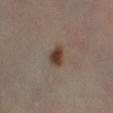The total-body-photography lesion software estimated internal color variation of about 4.5 on a 0–10 scale and peripheral color asymmetry of about 1.5. The analysis additionally found a classifier nevus-likeness of about 100/100 and a lesion-detection confidence of about 100/100. Located on the leg. Imaged with cross-polarized lighting. A roughly 15 mm field-of-view crop from a total-body skin photograph. The subject is a male aged around 65.